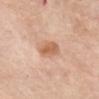Q: Was this lesion biopsied?
A: imaged on a skin check; not biopsied
Q: What did automated image analysis measure?
A: an automated nevus-likeness rating near 65 out of 100
Q: How was this image acquired?
A: total-body-photography crop, ~15 mm field of view
Q: Where on the body is the lesion?
A: the chest
Q: Lesion size?
A: ~3.5 mm (longest diameter)
Q: What are the patient's age and sex?
A: female, about 65 years old